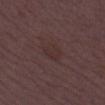{"biopsy_status": "not biopsied; imaged during a skin examination", "patient": {"sex": "female", "age_approx": 30}, "image": {"source": "total-body photography crop", "field_of_view_mm": 15}, "lesion_size": {"long_diameter_mm_approx": 3.0}, "automated_metrics": {"vs_skin_darker_L": 4.0}, "site": "left thigh", "lighting": "white-light"}